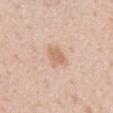Impression:
No biopsy was performed on this lesion — it was imaged during a full skin examination and was not determined to be concerning.
Image and clinical context:
The recorded lesion diameter is about 2.5 mm. Imaged with white-light lighting. The subject is a male aged 53–57. A 15 mm crop from a total-body photograph taken for skin-cancer surveillance. The lesion is located on the mid back.